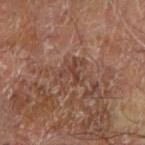Q: Is there a histopathology result?
A: total-body-photography surveillance lesion; no biopsy
Q: What is the lesion's diameter?
A: ~3 mm (longest diameter)
Q: What is the imaging modality?
A: total-body-photography crop, ~15 mm field of view
Q: What did automated image analysis measure?
A: a lesion–skin lightness drop of about 8 and a normalized border contrast of about 6.5; a border-irregularity index near 9.5/10, a color-variation rating of about 0/10, and radial color variation of about 0; a classifier nevus-likeness of about 0/100 and lesion-presence confidence of about 55/100
Q: What are the patient's age and sex?
A: male, about 65 years old
Q: What is the anatomic site?
A: the arm